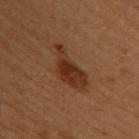Assessment:
Part of a total-body skin-imaging series; this lesion was reviewed on a skin check and was not flagged for biopsy.
Image and clinical context:
Located on the upper back. Longest diameter approximately 5.5 mm. A female subject aged approximately 50. Cropped from a whole-body photographic skin survey; the tile spans about 15 mm. An algorithmic analysis of the crop reported a lesion–skin lightness drop of about 8 and a normalized border contrast of about 8.5. This is a cross-polarized tile.